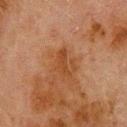Q: Was this lesion biopsied?
A: no biopsy performed (imaged during a skin exam)
Q: Automated lesion metrics?
A: an area of roughly 4.5 mm², a shape eccentricity near 0.9, and a shape-asymmetry score of about 0.4 (0 = symmetric); a border-irregularity index near 4.5/10, a within-lesion color-variation index near 1.5/10, and a peripheral color-asymmetry measure near 0.5; a classifier nevus-likeness of about 0/100 and a lesion-detection confidence of about 100/100
Q: Who is the patient?
A: male, in their 80s
Q: What kind of image is this?
A: ~15 mm crop, total-body skin-cancer survey
Q: Lesion location?
A: the upper back
Q: Illumination type?
A: cross-polarized